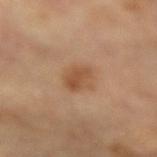Q: Was a biopsy performed?
A: total-body-photography surveillance lesion; no biopsy
Q: What kind of image is this?
A: total-body-photography crop, ~15 mm field of view
Q: Lesion size?
A: ≈3 mm
Q: How was the tile lit?
A: cross-polarized
Q: Where on the body is the lesion?
A: the left forearm
Q: Patient demographics?
A: female, aged approximately 65